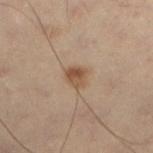Cropped from a whole-body photographic skin survey; the tile spans about 15 mm. The lesion is located on the leg. A male patient approximately 55 years of age.